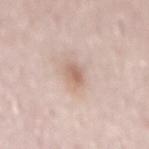  biopsy_status: not biopsied; imaged during a skin examination
  site: mid back
  lighting: white-light
  lesion_size:
    long_diameter_mm_approx: 2.5
  patient:
    sex: female
    age_approx: 50
  image:
    source: total-body photography crop
    field_of_view_mm: 15